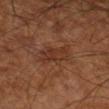Part of a total-body skin-imaging series; this lesion was reviewed on a skin check and was not flagged for biopsy.
A subject aged around 65.
The lesion's longest dimension is about 4 mm.
The lesion-visualizer software estimated an outline eccentricity of about 0.75 (0 = round, 1 = elongated) and a shape-asymmetry score of about 0.3 (0 = symmetric). The analysis additionally found an average lesion color of about L≈33 a*≈21 b*≈29 (CIELAB) and a lesion–skin lightness drop of about 6. And it measured a border-irregularity index near 3.5/10. And it measured an automated nevus-likeness rating near 0 out of 100.
Cropped from a total-body skin-imaging series; the visible field is about 15 mm.
The lesion is located on the right lower leg.